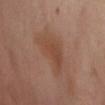  biopsy_status: not biopsied; imaged during a skin examination
  lesion_size:
    long_diameter_mm_approx: 5.0
  patient:
    sex: female
    age_approx: 55
  site: front of the torso
  image:
    source: total-body photography crop
    field_of_view_mm: 15